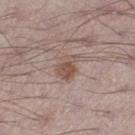Part of a total-body skin-imaging series; this lesion was reviewed on a skin check and was not flagged for biopsy.
A 15 mm close-up tile from a total-body photography series done for melanoma screening.
From the right thigh.
Imaged with white-light lighting.
A male subject, in their 70s.